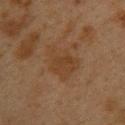Q: Was a biopsy performed?
A: imaged on a skin check; not biopsied
Q: How was this image acquired?
A: ~15 mm tile from a whole-body skin photo
Q: What is the lesion's diameter?
A: about 4.5 mm
Q: What are the patient's age and sex?
A: female, aged around 40
Q: Automated lesion metrics?
A: a lesion area of about 11 mm², an outline eccentricity of about 0.65 (0 = round, 1 = elongated), and a shape-asymmetry score of about 0.4 (0 = symmetric); a lesion color around L≈32 a*≈15 b*≈27 in CIELAB and a lesion–skin lightness drop of about 5
Q: Where on the body is the lesion?
A: the upper back
Q: How was the tile lit?
A: cross-polarized illumination